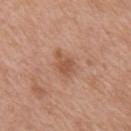Assessment: Part of a total-body skin-imaging series; this lesion was reviewed on a skin check and was not flagged for biopsy. Background: A lesion tile, about 15 mm wide, cut from a 3D total-body photograph. The lesion's longest dimension is about 3 mm. The patient is a male about 50 years old. Imaged with white-light lighting. Located on the mid back.